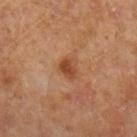Imaged during a routine full-body skin examination; the lesion was not biopsied and no histopathology is available.
A female patient, roughly 40 years of age.
A region of skin cropped from a whole-body photographic capture, roughly 15 mm wide.
Located on the left forearm.